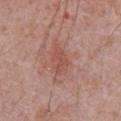Imaged with white-light lighting. Automated image analysis of the tile measured a footprint of about 4.5 mm², an outline eccentricity of about 0.85 (0 = round, 1 = elongated), and a symmetry-axis asymmetry near 0.45. It also reported border irregularity of about 4.5 on a 0–10 scale and a within-lesion color-variation index near 1/10. And it measured lesion-presence confidence of about 100/100. A roughly 15 mm field-of-view crop from a total-body skin photograph. The recorded lesion diameter is about 3.5 mm. On the chest. The patient is a male aged approximately 70.